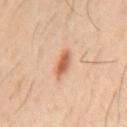<tbp_lesion>
  <biopsy_status>not biopsied; imaged during a skin examination</biopsy_status>
  <image>
    <source>total-body photography crop</source>
    <field_of_view_mm>15</field_of_view_mm>
  </image>
  <patient>
    <sex>male</sex>
    <age_approx>40</age_approx>
  </patient>
  <site>back</site>
</tbp_lesion>